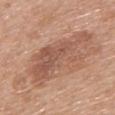No biopsy was performed on this lesion — it was imaged during a full skin examination and was not determined to be concerning. An algorithmic analysis of the crop reported a lesion-detection confidence of about 100/100. A region of skin cropped from a whole-body photographic capture, roughly 15 mm wide. The lesion is on the back. This is a white-light tile. A female subject, in their mid-60s. The lesion's longest dimension is about 8.5 mm.